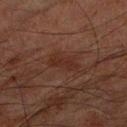Q: Is there a histopathology result?
A: no biopsy performed (imaged during a skin exam)
Q: What is the anatomic site?
A: the right forearm
Q: Lesion size?
A: about 3.5 mm
Q: Automated lesion metrics?
A: a footprint of about 3.5 mm² and a shape-asymmetry score of about 0.35 (0 = symmetric); a mean CIELAB color near L≈21 a*≈17 b*≈20, a lesion–skin lightness drop of about 5, and a normalized border contrast of about 6.5; a classifier nevus-likeness of about 15/100
Q: What is the imaging modality?
A: ~15 mm crop, total-body skin-cancer survey
Q: What are the patient's age and sex?
A: male, roughly 80 years of age